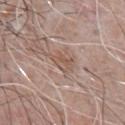notes: no biopsy performed (imaged during a skin exam) | patient: male, roughly 65 years of age | TBP lesion metrics: a lesion area of about 4 mm², an eccentricity of roughly 0.65, and a shape-asymmetry score of about 0.3 (0 = symmetric); roughly 7 lightness units darker than nearby skin and a normalized lesion–skin contrast near 6 | tile lighting: white-light | size: about 2.5 mm | anatomic site: the chest | image source: ~15 mm crop, total-body skin-cancer survey.